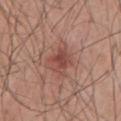No biopsy was performed on this lesion — it was imaged during a full skin examination and was not determined to be concerning.
This is a white-light tile.
The subject is a male in their mid-50s.
Measured at roughly 3 mm in maximum diameter.
Cropped from a total-body skin-imaging series; the visible field is about 15 mm.
On the chest.